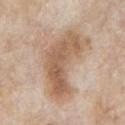Clinical impression:
Recorded during total-body skin imaging; not selected for excision or biopsy.
Image and clinical context:
On the chest. A close-up tile cropped from a whole-body skin photograph, about 15 mm across. A male patient roughly 65 years of age.